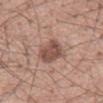Q: Was this lesion biopsied?
A: catalogued during a skin exam; not biopsied
Q: What is the imaging modality?
A: ~15 mm tile from a whole-body skin photo
Q: What are the patient's age and sex?
A: male, aged 53 to 57
Q: What is the anatomic site?
A: the mid back